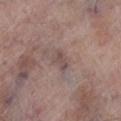Impression: The lesion was tiled from a total-body skin photograph and was not biopsied. Image and clinical context: Cropped from a total-body skin-imaging series; the visible field is about 15 mm. The subject is a male approximately 70 years of age. This is a white-light tile. An algorithmic analysis of the crop reported an average lesion color of about L≈48 a*≈16 b*≈18 (CIELAB) and a normalized border contrast of about 6. The lesion's longest dimension is about 2.5 mm. Located on the left lower leg.